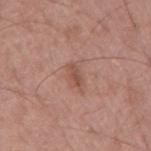Clinical impression:
The lesion was photographed on a routine skin check and not biopsied; there is no pathology result.
Context:
A lesion tile, about 15 mm wide, cut from a 3D total-body photograph. Located on the mid back. A male subject, roughly 60 years of age.